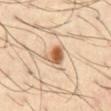Impression:
No biopsy was performed on this lesion — it was imaged during a full skin examination and was not determined to be concerning.
Context:
The lesion's longest dimension is about 3 mm. A close-up tile cropped from a whole-body skin photograph, about 15 mm across. On the front of the torso. A male patient approximately 40 years of age. This is a cross-polarized tile. An algorithmic analysis of the crop reported a footprint of about 6 mm² and a shape-asymmetry score of about 0.35 (0 = symmetric). The software also gave an average lesion color of about L≈60 a*≈20 b*≈35 (CIELAB) and about 15 CIELAB-L* units darker than the surrounding skin. It also reported internal color variation of about 9.5 on a 0–10 scale.